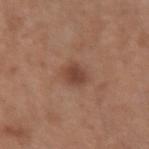follow-up = catalogued during a skin exam; not biopsied | image = ~15 mm tile from a whole-body skin photo | anatomic site = the right forearm | tile lighting = white-light illumination | subject = female, approximately 30 years of age.